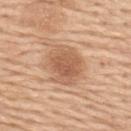workup = imaged on a skin check; not biopsied | body site = the upper back | tile lighting = white-light illumination | image source = ~15 mm tile from a whole-body skin photo | subject = female, aged 58 to 62 | size = ~4.5 mm (longest diameter).